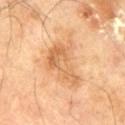workup: total-body-photography surveillance lesion; no biopsy | body site: the left thigh | image source: ~15 mm tile from a whole-body skin photo | patient: male, aged approximately 70.